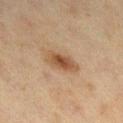No biopsy was performed on this lesion — it was imaged during a full skin examination and was not determined to be concerning. Captured under cross-polarized illumination. About 4 mm across. From the right lower leg. The total-body-photography lesion software estimated internal color variation of about 4.5 on a 0–10 scale and a peripheral color-asymmetry measure near 1. The software also gave a nevus-likeness score of about 90/100 and a lesion-detection confidence of about 100/100. A 15 mm crop from a total-body photograph taken for skin-cancer surveillance. The subject is a female about 40 years old.